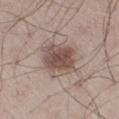Recorded during total-body skin imaging; not selected for excision or biopsy. A 15 mm crop from a total-body photograph taken for skin-cancer surveillance. Located on the right thigh. A male subject aged around 55.